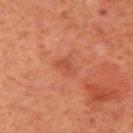  biopsy_status: not biopsied; imaged during a skin examination
  patient:
    sex: female
    age_approx: 40
  lighting: cross-polarized
  automated_metrics:
    cielab_L: 52
    cielab_a: 33
    cielab_b: 35
    vs_skin_darker_L: 7.0
    vs_skin_contrast_norm: 5.0
    nevus_likeness_0_100: 5
    lesion_detection_confidence_0_100: 100
  image:
    source: total-body photography crop
    field_of_view_mm: 15
  lesion_size:
    long_diameter_mm_approx: 2.5
  site: left upper arm